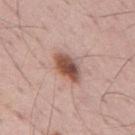This lesion was catalogued during total-body skin photography and was not selected for biopsy.
Captured under white-light illumination.
Cropped from a whole-body photographic skin survey; the tile spans about 15 mm.
About 4.5 mm across.
A male patient aged 68 to 72.
From the mid back.